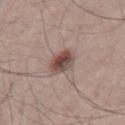{"biopsy_status": "not biopsied; imaged during a skin examination", "site": "mid back", "lesion_size": {"long_diameter_mm_approx": 3.5}, "image": {"source": "total-body photography crop", "field_of_view_mm": 15}, "lighting": "white-light", "patient": {"sex": "male", "age_approx": 35}}